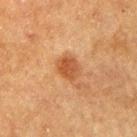Findings:
* notes — no biopsy performed (imaged during a skin exam)
* lesion size — ≈3 mm
* lighting — cross-polarized
* subject — male, in their mid-70s
* image — total-body-photography crop, ~15 mm field of view
* body site — the right upper arm
* automated lesion analysis — a footprint of about 5.5 mm², a shape eccentricity near 0.65, and a shape-asymmetry score of about 0.2 (0 = symmetric); a mean CIELAB color near L≈43 a*≈22 b*≈34, a lesion–skin lightness drop of about 9, and a normalized lesion–skin contrast near 7.5; a border-irregularity index near 2/10 and a color-variation rating of about 3/10; a nevus-likeness score of about 95/100